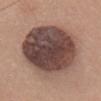Context: The patient is a female aged 33 to 37. The lesion is on the head or neck. Captured under white-light illumination. The lesion's longest dimension is about 9.5 mm. Cropped from a whole-body photographic skin survey; the tile spans about 15 mm.